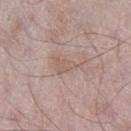| feature | finding |
|---|---|
| workup | total-body-photography surveillance lesion; no biopsy |
| acquisition | ~15 mm crop, total-body skin-cancer survey |
| site | the leg |
| patient | male, about 50 years old |
| lesion size | about 3.5 mm |